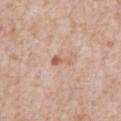Clinical impression: No biopsy was performed on this lesion — it was imaged during a full skin examination and was not determined to be concerning. Background: A male subject, roughly 80 years of age. From the chest. An algorithmic analysis of the crop reported a footprint of about 2.5 mm². The software also gave a lesion color around L≈61 a*≈20 b*≈31 in CIELAB, a lesion–skin lightness drop of about 9, and a normalized lesion–skin contrast near 6.5. It also reported border irregularity of about 4.5 on a 0–10 scale, a color-variation rating of about 0/10, and peripheral color asymmetry of about 0. And it measured a lesion-detection confidence of about 100/100. Captured under white-light illumination. About 2.5 mm across. A region of skin cropped from a whole-body photographic capture, roughly 15 mm wide.